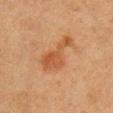follow-up: imaged on a skin check; not biopsied | size: about 5.5 mm | image source: 15 mm crop, total-body photography | subject: female, aged approximately 40 | lighting: cross-polarized illumination | body site: the right upper arm | TBP lesion metrics: an outline eccentricity of about 0.9 (0 = round, 1 = elongated) and a shape-asymmetry score of about 0.55 (0 = symmetric); about 8 CIELAB-L* units darker than the surrounding skin and a normalized lesion–skin contrast near 6.5; a nevus-likeness score of about 5/100.